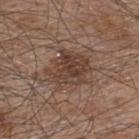The lesion was tiled from a total-body skin photograph and was not biopsied.
The tile uses white-light illumination.
A male subject about 45 years old.
A roughly 15 mm field-of-view crop from a total-body skin photograph.
The lesion is on the upper back.